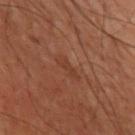Part of a total-body skin-imaging series; this lesion was reviewed on a skin check and was not flagged for biopsy.
The recorded lesion diameter is about 2.5 mm.
The tile uses cross-polarized illumination.
A region of skin cropped from a whole-body photographic capture, roughly 15 mm wide.
The subject is a male in their 70s.
From the arm.
The lesion-visualizer software estimated an outline eccentricity of about 0.9 (0 = round, 1 = elongated) and a symmetry-axis asymmetry near 0.25. It also reported a lesion color around L≈28 a*≈19 b*≈24 in CIELAB, roughly 4 lightness units darker than nearby skin, and a normalized lesion–skin contrast near 4.5. The software also gave border irregularity of about 3 on a 0–10 scale, a color-variation rating of about 0/10, and peripheral color asymmetry of about 0.